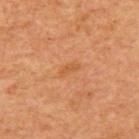The lesion was photographed on a routine skin check and not biopsied; there is no pathology result. From the chest. The tile uses cross-polarized illumination. A 15 mm close-up extracted from a 3D total-body photography capture. Approximately 2.5 mm at its widest. A male patient, aged approximately 60.